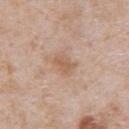| field | value |
|---|---|
| follow-up | catalogued during a skin exam; not biopsied |
| lighting | white-light |
| image source | ~15 mm crop, total-body skin-cancer survey |
| site | the abdomen |
| patient | male, in their mid- to late 60s |